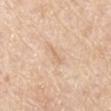Q: Is there a histopathology result?
A: no biopsy performed (imaged during a skin exam)
Q: What is the imaging modality?
A: ~15 mm tile from a whole-body skin photo
Q: Illumination type?
A: white-light illumination
Q: Who is the patient?
A: male, aged around 80
Q: Lesion location?
A: the left upper arm
Q: What is the lesion's diameter?
A: ~2.5 mm (longest diameter)